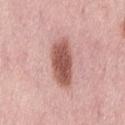{
  "biopsy_status": "not biopsied; imaged during a skin examination",
  "patient": {
    "sex": "female",
    "age_approx": 50
  },
  "automated_metrics": {
    "cielab_L": 56,
    "cielab_a": 24,
    "cielab_b": 25,
    "border_irregularity_0_10": 2.5,
    "color_variation_0_10": 4.0,
    "peripheral_color_asymmetry": 1.5
  },
  "site": "lower back",
  "lighting": "white-light",
  "image": {
    "source": "total-body photography crop",
    "field_of_view_mm": 15
  }
}